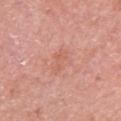Assessment:
The lesion was tiled from a total-body skin photograph and was not biopsied.
Acquisition and patient details:
Longest diameter approximately 2.5 mm. A female subject aged 63 to 67. Imaged with white-light lighting. The lesion is located on the arm. Cropped from a total-body skin-imaging series; the visible field is about 15 mm.